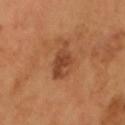Part of a total-body skin-imaging series; this lesion was reviewed on a skin check and was not flagged for biopsy.
On the head or neck.
Cropped from a whole-body photographic skin survey; the tile spans about 15 mm.
A male subject roughly 45 years of age.
The tile uses cross-polarized illumination.